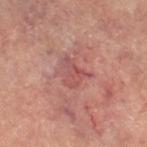Imaged during a routine full-body skin examination; the lesion was not biopsied and no histopathology is available.
A female subject, aged around 60.
Imaged with cross-polarized lighting.
A close-up tile cropped from a whole-body skin photograph, about 15 mm across.
Automated image analysis of the tile measured a lesion area of about 5.5 mm², an outline eccentricity of about 0.65 (0 = round, 1 = elongated), and a shape-asymmetry score of about 0.65 (0 = symmetric). The analysis additionally found a mean CIELAB color near L≈53 a*≈28 b*≈24, about 7 CIELAB-L* units darker than the surrounding skin, and a normalized lesion–skin contrast near 5.5. The software also gave a classifier nevus-likeness of about 0/100 and a lesion-detection confidence of about 95/100.
The lesion is located on the leg.